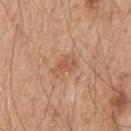Clinical impression: This lesion was catalogued during total-body skin photography and was not selected for biopsy. Context: Automated image analysis of the tile measured a lesion area of about 4 mm². A male subject, roughly 50 years of age. This image is a 15 mm lesion crop taken from a total-body photograph. Located on the left upper arm. The lesion's longest dimension is about 2.5 mm. The tile uses white-light illumination.